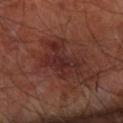<case>
  <biopsy_status>not biopsied; imaged during a skin examination</biopsy_status>
  <image>
    <source>total-body photography crop</source>
    <field_of_view_mm>15</field_of_view_mm>
  </image>
  <patient>
    <sex>male</sex>
    <age_approx>65</age_approx>
  </patient>
  <lighting>cross-polarized</lighting>
  <site>right forearm</site>
</case>